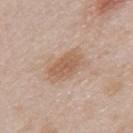From the upper back. A male subject, aged 38–42. A 15 mm close-up extracted from a 3D total-body photography capture. The lesion-visualizer software estimated a within-lesion color-variation index near 3/10. Approximately 4.5 mm at its widest. Imaged with white-light lighting.